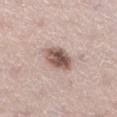notes: imaged on a skin check; not biopsied
acquisition: total-body-photography crop, ~15 mm field of view
location: the left lower leg
lesion diameter: ≈3.5 mm
TBP lesion metrics: an eccentricity of roughly 0.55 and two-axis asymmetry of about 0.1; border irregularity of about 1 on a 0–10 scale, internal color variation of about 5.5 on a 0–10 scale, and radial color variation of about 2; an automated nevus-likeness rating near 70 out of 100 and a detector confidence of about 100 out of 100 that the crop contains a lesion
subject: female, roughly 40 years of age